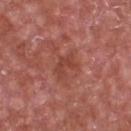biopsy status: total-body-photography surveillance lesion; no biopsy
patient: male, aged 63–67
diameter: ≈3 mm
image: ~15 mm tile from a whole-body skin photo
lighting: white-light illumination
location: the front of the torso